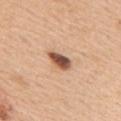Q: Is there a histopathology result?
A: total-body-photography surveillance lesion; no biopsy
Q: What lighting was used for the tile?
A: white-light
Q: Patient demographics?
A: male, in their 70s
Q: Where on the body is the lesion?
A: the mid back
Q: How was this image acquired?
A: ~15 mm crop, total-body skin-cancer survey